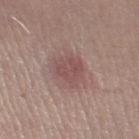notes: total-body-photography surveillance lesion; no biopsy
body site: the leg
image: total-body-photography crop, ~15 mm field of view
subject: female, roughly 20 years of age